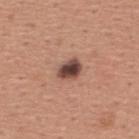workup: no biopsy performed (imaged during a skin exam)
automated lesion analysis: a lesion area of about 6 mm², a shape eccentricity near 0.7, and a shape-asymmetry score of about 0.2 (0 = symmetric)
illumination: white-light
anatomic site: the upper back
subject: male, in their mid-40s
imaging modality: ~15 mm tile from a whole-body skin photo